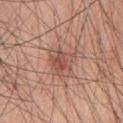Clinical impression:
No biopsy was performed on this lesion — it was imaged during a full skin examination and was not determined to be concerning.
Clinical summary:
The lesion is located on the chest. The patient is a male aged 58–62. A roughly 15 mm field-of-view crop from a total-body skin photograph. The lesion-visualizer software estimated an average lesion color of about L≈52 a*≈23 b*≈27 (CIELAB), a lesion–skin lightness drop of about 9, and a lesion-to-skin contrast of about 6.5 (normalized; higher = more distinct).